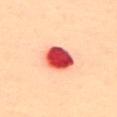Q: Is there a histopathology result?
A: catalogued during a skin exam; not biopsied
Q: How was this image acquired?
A: ~15 mm crop, total-body skin-cancer survey
Q: What lighting was used for the tile?
A: cross-polarized
Q: What did automated image analysis measure?
A: a lesion color around L≈48 a*≈43 b*≈32 in CIELAB, roughly 25 lightness units darker than nearby skin, and a lesion-to-skin contrast of about 15.5 (normalized; higher = more distinct); internal color variation of about 7 on a 0–10 scale and peripheral color asymmetry of about 2.5
Q: What is the anatomic site?
A: the upper back
Q: Who is the patient?
A: female, aged approximately 60